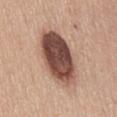No biopsy was performed on this lesion — it was imaged during a full skin examination and was not determined to be concerning.
Approximately 8 mm at its widest.
Imaged with white-light lighting.
Located on the front of the torso.
A lesion tile, about 15 mm wide, cut from a 3D total-body photograph.
The total-body-photography lesion software estimated a border-irregularity rating of about 1.5/10, a within-lesion color-variation index near 7/10, and peripheral color asymmetry of about 2.5.
The patient is a female aged 53–57.